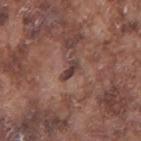Notes:
- follow-up: catalogued during a skin exam; not biopsied
- automated metrics: an area of roughly 3 mm², an eccentricity of roughly 0.9, and a symmetry-axis asymmetry near 0.4; an average lesion color of about L≈39 a*≈18 b*≈20 (CIELAB), roughly 11 lightness units darker than nearby skin, and a normalized border contrast of about 9.5; internal color variation of about 0.5 on a 0–10 scale
- subject: male, approximately 75 years of age
- anatomic site: the right upper arm
- illumination: white-light
- image source: 15 mm crop, total-body photography